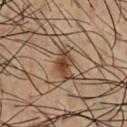| key | value |
|---|---|
| biopsy status | catalogued during a skin exam; not biopsied |
| lesion diameter | ~2.5 mm (longest diameter) |
| subject | male, in their mid-50s |
| lighting | cross-polarized |
| anatomic site | the chest |
| image source | 15 mm crop, total-body photography |